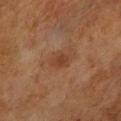A male subject aged 68 to 72. Automated tile analysis of the lesion measured an area of roughly 4 mm², a shape eccentricity near 0.75, and a shape-asymmetry score of about 0.15 (0 = symmetric). It also reported a nevus-likeness score of about 35/100 and a lesion-detection confidence of about 100/100. This is a cross-polarized tile. The lesion is on the right lower leg. A 15 mm crop from a total-body photograph taken for skin-cancer surveillance. Approximately 2.5 mm at its widest.